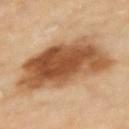The lesion was tiled from a total-body skin photograph and was not biopsied. A roughly 15 mm field-of-view crop from a total-body skin photograph. Captured under cross-polarized illumination. Automated image analysis of the tile measured an outline eccentricity of about 0.7 (0 = round, 1 = elongated) and a shape-asymmetry score of about 0.2 (0 = symmetric). And it measured a border-irregularity rating of about 2.5/10 and a peripheral color-asymmetry measure near 2. The software also gave an automated nevus-likeness rating near 90 out of 100 and a lesion-detection confidence of about 100/100. The lesion is located on the left upper arm. About 9.5 mm across. The patient is a female roughly 60 years of age.